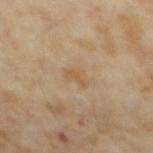Impression: No biopsy was performed on this lesion — it was imaged during a full skin examination and was not determined to be concerning. Acquisition and patient details: Captured under cross-polarized illumination. A 15 mm crop from a total-body photograph taken for skin-cancer surveillance. A male patient, approximately 65 years of age. About 2.5 mm across.